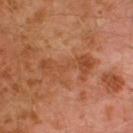No biopsy was performed on this lesion — it was imaged during a full skin examination and was not determined to be concerning. Longest diameter approximately 6.5 mm. Automated tile analysis of the lesion measured a lesion area of about 11 mm², an eccentricity of roughly 0.95, and two-axis asymmetry of about 0.4. And it measured a border-irregularity index near 7.5/10, a color-variation rating of about 5/10, and peripheral color asymmetry of about 1.5. The analysis additionally found an automated nevus-likeness rating near 0 out of 100. The tile uses cross-polarized illumination. A male subject aged around 30. A roughly 15 mm field-of-view crop from a total-body skin photograph. Located on the left lower leg.